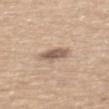{
  "biopsy_status": "not biopsied; imaged during a skin examination",
  "patient": {
    "sex": "male",
    "age_approx": 65
  },
  "site": "mid back",
  "image": {
    "source": "total-body photography crop",
    "field_of_view_mm": 15
  }
}